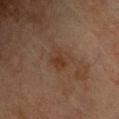This lesion was catalogued during total-body skin photography and was not selected for biopsy. This image is a 15 mm lesion crop taken from a total-body photograph. Approximately 3 mm at its widest. The patient is a male aged approximately 75. An algorithmic analysis of the crop reported a lesion area of about 5.5 mm², an outline eccentricity of about 0.6 (0 = round, 1 = elongated), and a shape-asymmetry score of about 0.2 (0 = symmetric). And it measured a border-irregularity index near 2/10, a within-lesion color-variation index near 3/10, and peripheral color asymmetry of about 1. The lesion is located on the chest. The tile uses cross-polarized illumination.